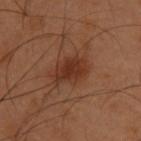| field | value |
|---|---|
| follow-up | catalogued during a skin exam; not biopsied |
| patient | male, aged 53 to 57 |
| site | the upper back |
| automated lesion analysis | a lesion color around L≈32 a*≈22 b*≈29 in CIELAB, about 8 CIELAB-L* units darker than the surrounding skin, and a normalized border contrast of about 7.5; a nevus-likeness score of about 95/100 and a lesion-detection confidence of about 100/100 |
| tile lighting | cross-polarized |
| image | ~15 mm crop, total-body skin-cancer survey |
| lesion size | ~4.5 mm (longest diameter) |